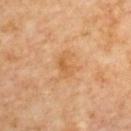Q: What is the anatomic site?
A: the upper back
Q: Lesion size?
A: about 3 mm
Q: Illumination type?
A: cross-polarized illumination
Q: What are the patient's age and sex?
A: female, about 65 years old
Q: How was this image acquired?
A: total-body-photography crop, ~15 mm field of view